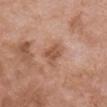Part of a total-body skin-imaging series; this lesion was reviewed on a skin check and was not flagged for biopsy.
The patient is a female in their 70s.
From the chest.
Captured under white-light illumination.
The recorded lesion diameter is about 2.5 mm.
The lesion-visualizer software estimated a border-irregularity rating of about 2.5/10 and a peripheral color-asymmetry measure near 0.5. And it measured a classifier nevus-likeness of about 10/100 and lesion-presence confidence of about 100/100.
A region of skin cropped from a whole-body photographic capture, roughly 15 mm wide.